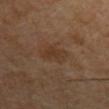This image is a 15 mm lesion crop taken from a total-body photograph. A male patient, approximately 45 years of age. The lesion-visualizer software estimated an area of roughly 7 mm², a shape eccentricity near 0.6, and a symmetry-axis asymmetry near 0.3. The analysis additionally found a border-irregularity index near 3/10, a within-lesion color-variation index near 2/10, and peripheral color asymmetry of about 0.5. And it measured a lesion-detection confidence of about 100/100. From the left upper arm.